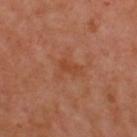Q: Was a biopsy performed?
A: total-body-photography surveillance lesion; no biopsy
Q: How was the tile lit?
A: cross-polarized
Q: Who is the patient?
A: male, approximately 50 years of age
Q: Lesion size?
A: ≈3 mm
Q: Where on the body is the lesion?
A: the back
Q: What did automated image analysis measure?
A: a lesion area of about 5.5 mm², an eccentricity of roughly 0.7, and two-axis asymmetry of about 0.5; a border-irregularity rating of about 5.5/10, a within-lesion color-variation index near 2/10, and radial color variation of about 0.5
Q: How was this image acquired?
A: total-body-photography crop, ~15 mm field of view